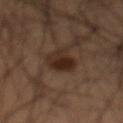No biopsy was performed on this lesion — it was imaged during a full skin examination and was not determined to be concerning. The lesion-visualizer software estimated a footprint of about 8 mm² and a symmetry-axis asymmetry near 0.25. It also reported a mean CIELAB color near L≈24 a*≈15 b*≈22, roughly 9 lightness units darker than nearby skin, and a lesion-to-skin contrast of about 10 (normalized; higher = more distinct). The software also gave lesion-presence confidence of about 100/100. This is a cross-polarized tile. A lesion tile, about 15 mm wide, cut from a 3D total-body photograph. From the abdomen. Longest diameter approximately 4 mm. The patient is a male in their mid-50s.